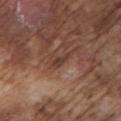<case>
<biopsy_status>not biopsied; imaged during a skin examination</biopsy_status>
<site>left upper arm</site>
<image>
  <source>total-body photography crop</source>
  <field_of_view_mm>15</field_of_view_mm>
</image>
<patient>
  <sex>male</sex>
  <age_approx>75</age_approx>
</patient>
</case>